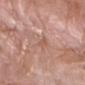Context: This is a white-light tile. The subject is a male aged around 60. This image is a 15 mm lesion crop taken from a total-body photograph. On the right forearm.